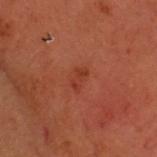Captured during whole-body skin photography for melanoma surveillance; the lesion was not biopsied.
From the head or neck.
Longest diameter approximately 3 mm.
The subject is a male in their 50s.
This image is a 15 mm lesion crop taken from a total-body photograph.